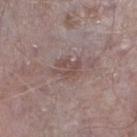Imaged during a routine full-body skin examination; the lesion was not biopsied and no histopathology is available. On the right lower leg. Captured under white-light illumination. A male patient, in their mid-70s. A region of skin cropped from a whole-body photographic capture, roughly 15 mm wide. The recorded lesion diameter is about 3.5 mm.